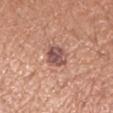Q: Was a biopsy performed?
A: total-body-photography surveillance lesion; no biopsy
Q: What kind of image is this?
A: ~15 mm crop, total-body skin-cancer survey
Q: Patient demographics?
A: male, approximately 55 years of age
Q: Automated lesion metrics?
A: an area of roughly 6 mm² and an outline eccentricity of about 0.4 (0 = round, 1 = elongated); an average lesion color of about L≈52 a*≈22 b*≈24 (CIELAB), roughly 12 lightness units darker than nearby skin, and a normalized border contrast of about 9; border irregularity of about 2 on a 0–10 scale, a color-variation rating of about 3.5/10, and peripheral color asymmetry of about 1
Q: What is the lesion's diameter?
A: ~2.5 mm (longest diameter)
Q: How was the tile lit?
A: white-light illumination
Q: Where on the body is the lesion?
A: the arm